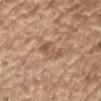Part of a total-body skin-imaging series; this lesion was reviewed on a skin check and was not flagged for biopsy. From the right upper arm. Cropped from a total-body skin-imaging series; the visible field is about 15 mm. This is a white-light tile. A male subject, roughly 70 years of age. About 4 mm across.